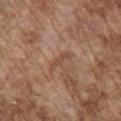{
  "biopsy_status": "not biopsied; imaged during a skin examination",
  "image": {
    "source": "total-body photography crop",
    "field_of_view_mm": 15
  },
  "lighting": "white-light",
  "automated_metrics": {
    "area_mm2_approx": 3.0,
    "eccentricity": 0.95,
    "shape_asymmetry": 0.35,
    "border_irregularity_0_10": 4.5,
    "color_variation_0_10": 0.0
  },
  "site": "chest",
  "patient": {
    "sex": "male",
    "age_approx": 75
  }
}